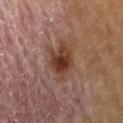Clinical impression:
The lesion was photographed on a routine skin check and not biopsied; there is no pathology result.
Clinical summary:
Cropped from a total-body skin-imaging series; the visible field is about 15 mm. The subject is a male about 85 years old or older. Located on the lower back.